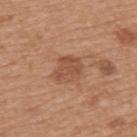Clinical impression: Part of a total-body skin-imaging series; this lesion was reviewed on a skin check and was not flagged for biopsy.